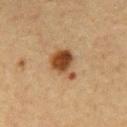Captured during whole-body skin photography for melanoma surveillance; the lesion was not biopsied. A male subject about 75 years old. The lesion is on the mid back. The lesion's longest dimension is about 4 mm. A close-up tile cropped from a whole-body skin photograph, about 15 mm across.